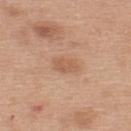Q: Was a biopsy performed?
A: catalogued during a skin exam; not biopsied
Q: What did automated image analysis measure?
A: an area of roughly 4 mm² and a symmetry-axis asymmetry near 0.25; a lesion color around L≈58 a*≈21 b*≈33 in CIELAB, about 8 CIELAB-L* units darker than the surrounding skin, and a normalized border contrast of about 5.5; a border-irregularity index near 2.5/10, internal color variation of about 2 on a 0–10 scale, and radial color variation of about 0.5; a nevus-likeness score of about 20/100
Q: Illumination type?
A: white-light illumination
Q: What kind of image is this?
A: ~15 mm tile from a whole-body skin photo
Q: What is the anatomic site?
A: the upper back
Q: Who is the patient?
A: female, aged 38–42
Q: Lesion size?
A: ~2.5 mm (longest diameter)